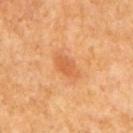Recorded during total-body skin imaging; not selected for excision or biopsy. A 15 mm close-up tile from a total-body photography series done for melanoma screening. Located on the right upper arm. Approximately 3 mm at its widest. This is a cross-polarized tile. A male subject, aged around 55.